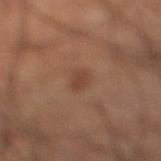Clinical impression:
No biopsy was performed on this lesion — it was imaged during a full skin examination and was not determined to be concerning.
Image and clinical context:
The subject is a male aged around 60. The tile uses cross-polarized illumination. A roughly 15 mm field-of-view crop from a total-body skin photograph. From the right lower leg.